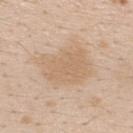Recorded during total-body skin imaging; not selected for excision or biopsy. A 15 mm crop from a total-body photograph taken for skin-cancer surveillance. A male subject, roughly 25 years of age. The tile uses white-light illumination. The lesion is located on the back. Automated image analysis of the tile measured a border-irregularity index near 3/10, a within-lesion color-variation index near 1.5/10, and peripheral color asymmetry of about 0.5. The lesion's longest dimension is about 6 mm.